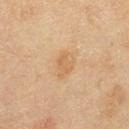This lesion was catalogued during total-body skin photography and was not selected for biopsy.
About 3 mm across.
A 15 mm close-up extracted from a 3D total-body photography capture.
The lesion is located on the left thigh.
Imaged with cross-polarized lighting.
The patient is a female approximately 60 years of age.